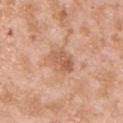{
  "biopsy_status": "not biopsied; imaged during a skin examination",
  "image": {
    "source": "total-body photography crop",
    "field_of_view_mm": 15
  },
  "automated_metrics": {
    "cielab_L": 59,
    "cielab_a": 22,
    "cielab_b": 33,
    "vs_skin_darker_L": 10.0,
    "vs_skin_contrast_norm": 6.5,
    "nevus_likeness_0_100": 5
  },
  "lighting": "white-light",
  "patient": {
    "sex": "male",
    "age_approx": 25
  },
  "site": "right upper arm",
  "lesion_size": {
    "long_diameter_mm_approx": 4.0
  }
}